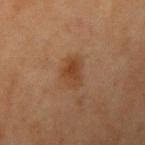Imaged during a routine full-body skin examination; the lesion was not biopsied and no histopathology is available. Automated image analysis of the tile measured about 7 CIELAB-L* units darker than the surrounding skin. The software also gave border irregularity of about 2.5 on a 0–10 scale and peripheral color asymmetry of about 1. It also reported an automated nevus-likeness rating near 60 out of 100 and lesion-presence confidence of about 100/100. Cropped from a whole-body photographic skin survey; the tile spans about 15 mm. Imaged with cross-polarized lighting. A female patient, about 40 years old. Located on the right upper arm.